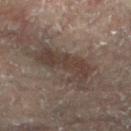- follow-up · total-body-photography surveillance lesion; no biopsy
- imaging modality · ~15 mm crop, total-body skin-cancer survey
- subject · female, approximately 80 years of age
- automated lesion analysis · an area of roughly 15 mm², an outline eccentricity of about 0.9 (0 = round, 1 = elongated), and two-axis asymmetry of about 0.45; about 8 CIELAB-L* units darker than the surrounding skin and a normalized lesion–skin contrast near 7.5; border irregularity of about 7 on a 0–10 scale and radial color variation of about 0.5
- anatomic site · the right leg
- size · ≈7 mm
- illumination · cross-polarized